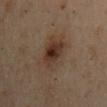A male patient roughly 55 years of age.
This image is a 15 mm lesion crop taken from a total-body photograph.
On the mid back.